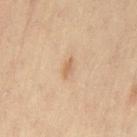follow-up: catalogued during a skin exam; not biopsied
lesion diameter: ≈2.5 mm
patient: female, aged around 35
anatomic site: the lower back
image source: 15 mm crop, total-body photography
lighting: cross-polarized illumination
TBP lesion metrics: a footprint of about 2.5 mm², an outline eccentricity of about 0.9 (0 = round, 1 = elongated), and a symmetry-axis asymmetry near 0.3; an average lesion color of about L≈61 a*≈18 b*≈34 (CIELAB) and roughly 8 lightness units darker than nearby skin; a border-irregularity index near 3/10, a within-lesion color-variation index near 0/10, and peripheral color asymmetry of about 0